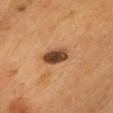Assessment: The lesion was tiled from a total-body skin photograph and was not biopsied. Background: The lesion is on the chest. A lesion tile, about 15 mm wide, cut from a 3D total-body photograph. A male patient roughly 65 years of age. Imaged with cross-polarized lighting.